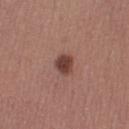The lesion was photographed on a routine skin check and not biopsied; there is no pathology result.
The patient is a female aged approximately 30.
A region of skin cropped from a whole-body photographic capture, roughly 15 mm wide.
On the left thigh.
Captured under white-light illumination.
About 2.5 mm across.